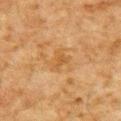follow-up — imaged on a skin check; not biopsied
patient — male, about 80 years old
body site — the upper back
lighting — cross-polarized
image source — 15 mm crop, total-body photography
lesion diameter — ~3 mm (longest diameter)